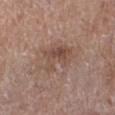Captured during whole-body skin photography for melanoma surveillance; the lesion was not biopsied. The lesion is on the left lower leg. Imaged with white-light lighting. This image is a 15 mm lesion crop taken from a total-body photograph. The recorded lesion diameter is about 4.5 mm. A female patient aged around 75.